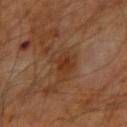biopsy status: no biopsy performed (imaged during a skin exam) | patient: male, about 60 years old | site: the left forearm | image source: ~15 mm tile from a whole-body skin photo | size: about 4.5 mm | illumination: cross-polarized.